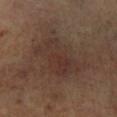This lesion was catalogued during total-body skin photography and was not selected for biopsy. The total-body-photography lesion software estimated a lesion area of about 19 mm², an outline eccentricity of about 0.75 (0 = round, 1 = elongated), and two-axis asymmetry of about 0.25. It also reported a lesion color around L≈32 a*≈16 b*≈21 in CIELAB, a lesion–skin lightness drop of about 5, and a normalized lesion–skin contrast near 5.5. It also reported a border-irregularity rating of about 3/10, a color-variation rating of about 3.5/10, and peripheral color asymmetry of about 1. It also reported a classifier nevus-likeness of about 0/100 and lesion-presence confidence of about 100/100. A male patient approximately 65 years of age. Cropped from a whole-body photographic skin survey; the tile spans about 15 mm. Longest diameter approximately 6 mm.